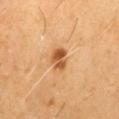Case summary:
• follow-up: total-body-photography surveillance lesion; no biopsy
• illumination: cross-polarized
• site: the right thigh
• image: ~15 mm crop, total-body skin-cancer survey
• diameter: about 2.5 mm
• patient: male, aged 58–62